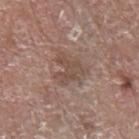Context:
From the left upper arm. A male patient, aged around 70. A lesion tile, about 15 mm wide, cut from a 3D total-body photograph. Automated image analysis of the tile measured a lesion area of about 8 mm², a shape eccentricity near 0.4, and a shape-asymmetry score of about 0.55 (0 = symmetric). It also reported an average lesion color of about L≈48 a*≈17 b*≈24 (CIELAB), about 7 CIELAB-L* units darker than the surrounding skin, and a normalized border contrast of about 5.5. And it measured a border-irregularity rating of about 7/10 and a color-variation rating of about 2/10. The software also gave a nevus-likeness score of about 0/100 and a detector confidence of about 100 out of 100 that the crop contains a lesion. Imaged with white-light lighting.